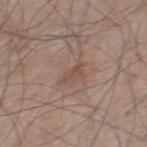Impression: Recorded during total-body skin imaging; not selected for excision or biopsy. Image and clinical context: Located on the right thigh. The tile uses white-light illumination. A 15 mm close-up tile from a total-body photography series done for melanoma screening. The patient is a male aged approximately 60. Longest diameter approximately 3 mm.